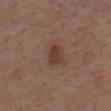Findings:
- follow-up — total-body-photography surveillance lesion; no biopsy
- diameter — ≈2.5 mm
- acquisition — 15 mm crop, total-body photography
- site — the back
- patient — male, aged approximately 70